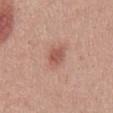follow-up=total-body-photography surveillance lesion; no biopsy
site=the mid back
automated lesion analysis=a within-lesion color-variation index near 2/10 and radial color variation of about 0.5; a nevus-likeness score of about 80/100
patient=male, about 30 years old
lesion size=~2.5 mm (longest diameter)
image source=~15 mm tile from a whole-body skin photo